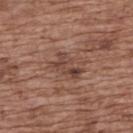biopsy status=catalogued during a skin exam; not biopsied